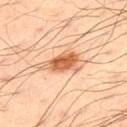Assessment: The lesion was tiled from a total-body skin photograph and was not biopsied. Clinical summary: A region of skin cropped from a whole-body photographic capture, roughly 15 mm wide. Imaged with cross-polarized lighting. On the back. Automated tile analysis of the lesion measured a lesion color around L≈64 a*≈25 b*≈39 in CIELAB and about 15 CIELAB-L* units darker than the surrounding skin. And it measured border irregularity of about 4 on a 0–10 scale, a within-lesion color-variation index near 6.5/10, and radial color variation of about 2. And it measured lesion-presence confidence of about 100/100. The patient is a male aged 48 to 52. Approximately 5.5 mm at its widest.